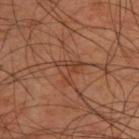The lesion was tiled from a total-body skin photograph and was not biopsied.
Longest diameter approximately 3 mm.
The subject is a male approximately 45 years of age.
A 15 mm crop from a total-body photograph taken for skin-cancer surveillance.
The tile uses cross-polarized illumination.
The lesion is on the upper back.